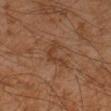biopsy status: no biopsy performed (imaged during a skin exam)
image-analysis metrics: an outline eccentricity of about 0.9 (0 = round, 1 = elongated); a lesion color around L≈31 a*≈17 b*≈26 in CIELAB, roughly 5 lightness units darker than nearby skin, and a normalized border contrast of about 5.5; a classifier nevus-likeness of about 0/100
diameter: about 5 mm
lighting: cross-polarized illumination
patient: male, aged 43–47
location: the arm
acquisition: 15 mm crop, total-body photography